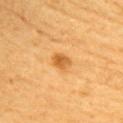Case summary:
• follow-up: no biopsy performed (imaged during a skin exam)
• tile lighting: cross-polarized illumination
• acquisition: ~15 mm crop, total-body skin-cancer survey
• subject: female, in their mid-60s
• lesion size: about 2.5 mm
• location: the upper back